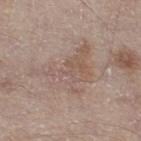This lesion was catalogued during total-body skin photography and was not selected for biopsy.
The patient is a male aged 63–67.
A region of skin cropped from a whole-body photographic capture, roughly 15 mm wide.
From the right thigh.